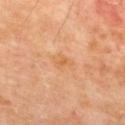Case summary:
• follow-up — imaged on a skin check; not biopsied
• body site — the back
• lesion diameter — about 3 mm
• subject — male, approximately 70 years of age
• imaging modality — total-body-photography crop, ~15 mm field of view
• lighting — cross-polarized illumination